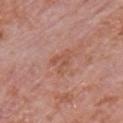{
  "automated_metrics": {
    "area_mm2_approx": 3.5,
    "shape_asymmetry": 0.55,
    "cielab_L": 53,
    "cielab_a": 25,
    "cielab_b": 30,
    "vs_skin_darker_L": 6.0,
    "vs_skin_contrast_norm": 5.5
  },
  "patient": {
    "sex": "male",
    "age_approx": 80
  },
  "site": "chest",
  "lesion_size": {
    "long_diameter_mm_approx": 2.5
  },
  "image": {
    "source": "total-body photography crop",
    "field_of_view_mm": 15
  },
  "lighting": "white-light"
}